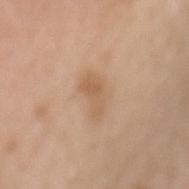notes = catalogued during a skin exam; not biopsied
size = ≈4 mm
subject = female, about 70 years old
illumination = white-light
image source = ~15 mm tile from a whole-body skin photo
automated metrics = a footprint of about 5.5 mm², a shape eccentricity near 0.9, and a symmetry-axis asymmetry near 0.4
anatomic site = the mid back